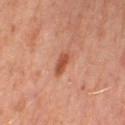patient: female, aged 48–52
imaging modality: total-body-photography crop, ~15 mm field of view
location: the leg